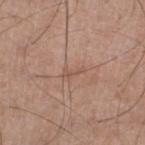{
  "biopsy_status": "not biopsied; imaged during a skin examination",
  "lighting": "white-light",
  "site": "left lower leg",
  "patient": {
    "sex": "male",
    "age_approx": 60
  },
  "image": {
    "source": "total-body photography crop",
    "field_of_view_mm": 15
  }
}